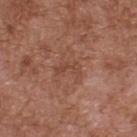Context: A male subject, aged around 75. On the upper back. Cropped from a whole-body photographic skin survey; the tile spans about 15 mm.